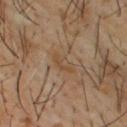{"automated_metrics": {"vs_skin_darker_L": 5.0, "vs_skin_contrast_norm": 5.5, "border_irregularity_0_10": 2.5, "color_variation_0_10": 2.0, "peripheral_color_asymmetry": 0.5, "nevus_likeness_0_100": 5, "lesion_detection_confidence_0_100": 75}, "patient": {"sex": "male", "age_approx": 65}, "site": "upper back", "image": {"source": "total-body photography crop", "field_of_view_mm": 15}, "lesion_size": {"long_diameter_mm_approx": 3.0}, "lighting": "cross-polarized"}